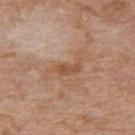Part of a total-body skin-imaging series; this lesion was reviewed on a skin check and was not flagged for biopsy.
Cropped from a total-body skin-imaging series; the visible field is about 15 mm.
On the upper back.
An algorithmic analysis of the crop reported a footprint of about 8 mm² and an outline eccentricity of about 0.9 (0 = round, 1 = elongated). It also reported an average lesion color of about L≈54 a*≈20 b*≈31 (CIELAB), about 8 CIELAB-L* units darker than the surrounding skin, and a lesion-to-skin contrast of about 6 (normalized; higher = more distinct). It also reported internal color variation of about 2.5 on a 0–10 scale and a peripheral color-asymmetry measure near 1.
Measured at roughly 5.5 mm in maximum diameter.
A female patient in their mid-70s.